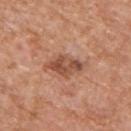Part of a total-body skin-imaging series; this lesion was reviewed on a skin check and was not flagged for biopsy. Located on the upper back. A 15 mm crop from a total-body photograph taken for skin-cancer surveillance. The subject is a male about 65 years old.